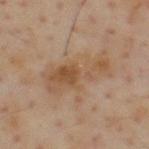Q: Was a biopsy performed?
A: catalogued during a skin exam; not biopsied
Q: What kind of image is this?
A: 15 mm crop, total-body photography
Q: Who is the patient?
A: male, roughly 55 years of age
Q: What is the anatomic site?
A: the upper back
Q: Lesion size?
A: about 7 mm